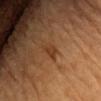Q: Is there a histopathology result?
A: no biopsy performed (imaged during a skin exam)
Q: What is the lesion's diameter?
A: ≈3 mm
Q: What did automated image analysis measure?
A: a mean CIELAB color near L≈32 a*≈20 b*≈30, roughly 6 lightness units darker than nearby skin, and a normalized border contrast of about 6; a border-irregularity rating of about 3.5/10 and internal color variation of about 2 on a 0–10 scale; a lesion-detection confidence of about 100/100
Q: What kind of image is this?
A: 15 mm crop, total-body photography
Q: Patient demographics?
A: male, in their mid- to late 80s or older
Q: Illumination type?
A: cross-polarized illumination
Q: Lesion location?
A: the chest